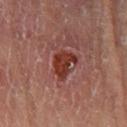Assessment:
The lesion was photographed on a routine skin check and not biopsied; there is no pathology result.
Image and clinical context:
A region of skin cropped from a whole-body photographic capture, roughly 15 mm wide. Approximately 3.5 mm at its widest. An algorithmic analysis of the crop reported a mean CIELAB color near L≈31 a*≈25 b*≈25, roughly 10 lightness units darker than nearby skin, and a normalized border contrast of about 10.5. The software also gave a classifier nevus-likeness of about 0/100 and a detector confidence of about 100 out of 100 that the crop contains a lesion. The tile uses cross-polarized illumination. The lesion is located on the left lower leg. The subject is a male approximately 55 years of age.